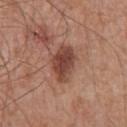Q: Was this lesion biopsied?
A: no biopsy performed (imaged during a skin exam)
Q: Automated lesion metrics?
A: a shape eccentricity near 0.8 and two-axis asymmetry of about 0.15; an average lesion color of about L≈44 a*≈23 b*≈27 (CIELAB) and a normalized lesion–skin contrast near 9.5; a color-variation rating of about 3.5/10
Q: What is the anatomic site?
A: the chest
Q: What are the patient's age and sex?
A: male, roughly 65 years of age
Q: What lighting was used for the tile?
A: white-light illumination
Q: What is the imaging modality?
A: total-body-photography crop, ~15 mm field of view